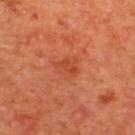| field | value |
|---|---|
| site | the upper back |
| patient | male, in their mid-60s |
| image source | ~15 mm tile from a whole-body skin photo |
| tile lighting | cross-polarized illumination |
| lesion diameter | about 3 mm |